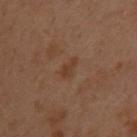The lesion was tiled from a total-body skin photograph and was not biopsied.
A female patient about 55 years old.
The recorded lesion diameter is about 2.5 mm.
This image is a 15 mm lesion crop taken from a total-body photograph.
This is a cross-polarized tile.
The lesion is on the upper back.